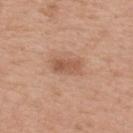• workup: total-body-photography surveillance lesion; no biopsy
• lesion size: ~3.5 mm (longest diameter)
• location: the upper back
• image: ~15 mm tile from a whole-body skin photo
• tile lighting: white-light illumination
• patient: male, aged 63 to 67